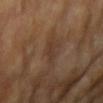A female patient, aged 73 to 77.
About 3 mm across.
A 15 mm close-up tile from a total-body photography series done for melanoma screening.
The lesion is located on the left upper arm.
Automated image analysis of the tile measured a footprint of about 4 mm² and an eccentricity of roughly 0.8. And it measured a lesion–skin lightness drop of about 5 and a normalized border contrast of about 5. It also reported a lesion-detection confidence of about 100/100.
Imaged with cross-polarized lighting.